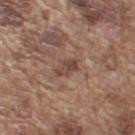<tbp_lesion>
  <automated_metrics>
    <cielab_L>45</cielab_L>
    <cielab_a>18</cielab_a>
    <cielab_b>24</cielab_b>
    <vs_skin_darker_L>9.0</vs_skin_darker_L>
    <vs_skin_contrast_norm>7.0</vs_skin_contrast_norm>
    <border_irregularity_0_10>3.0</border_irregularity_0_10>
    <color_variation_0_10>3.0</color_variation_0_10>
    <peripheral_color_asymmetry>1.5</peripheral_color_asymmetry>
  </automated_metrics>
  <patient>
    <sex>male</sex>
    <age_approx>75</age_approx>
  </patient>
  <lighting>white-light</lighting>
  <image>
    <source>total-body photography crop</source>
    <field_of_view_mm>15</field_of_view_mm>
  </image>
  <site>arm</site>
  <lesion_size>
    <long_diameter_mm_approx>3.0</long_diameter_mm_approx>
  </lesion_size>
</tbp_lesion>